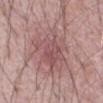| key | value |
|---|---|
| tile lighting | white-light |
| size | ~6 mm (longest diameter) |
| subject | male, aged 68 to 72 |
| location | the abdomen |
| acquisition | ~15 mm crop, total-body skin-cancer survey |